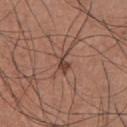No biopsy was performed on this lesion — it was imaged during a full skin examination and was not determined to be concerning. Captured under white-light illumination. A close-up tile cropped from a whole-body skin photograph, about 15 mm across. The patient is a male about 35 years old. Located on the front of the torso.